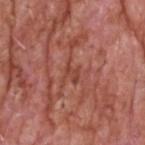Q: Is there a histopathology result?
A: no biopsy performed (imaged during a skin exam)
Q: How was this image acquired?
A: total-body-photography crop, ~15 mm field of view
Q: What is the anatomic site?
A: the head or neck
Q: How large is the lesion?
A: ~2.5 mm (longest diameter)
Q: What are the patient's age and sex?
A: male, aged 58 to 62
Q: What lighting was used for the tile?
A: white-light